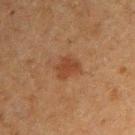<lesion>
  <biopsy_status>not biopsied; imaged during a skin examination</biopsy_status>
  <image>
    <source>total-body photography crop</source>
    <field_of_view_mm>15</field_of_view_mm>
  </image>
  <site>right upper arm</site>
  <lesion_size>
    <long_diameter_mm_approx>3.0</long_diameter_mm_approx>
  </lesion_size>
  <patient>
    <sex>male</sex>
    <age_approx>50</age_approx>
  </patient>
  <lighting>cross-polarized</lighting>
</lesion>